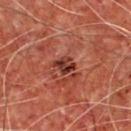Findings:
* follow-up · imaged on a skin check; not biopsied
* illumination · cross-polarized
* image-analysis metrics · a lesion area of about 4 mm², an eccentricity of roughly 0.5, and a symmetry-axis asymmetry near 0.2; a mean CIELAB color near L≈32 a*≈26 b*≈26, roughly 11 lightness units darker than nearby skin, and a lesion-to-skin contrast of about 10 (normalized; higher = more distinct); a border-irregularity rating of about 2/10 and a color-variation rating of about 6.5/10
* location · the chest
* subject · male, aged 63 to 67
* diameter · about 2.5 mm
* image source · total-body-photography crop, ~15 mm field of view